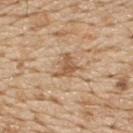notes — total-body-photography surveillance lesion; no biopsy
image-analysis metrics — an outline eccentricity of about 0.65 (0 = round, 1 = elongated)
acquisition — total-body-photography crop, ~15 mm field of view
lighting — white-light illumination
patient — male, aged around 70
anatomic site — the upper back
lesion size — about 3 mm